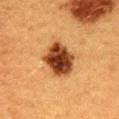The lesion was tiled from a total-body skin photograph and was not biopsied. Automated tile analysis of the lesion measured a lesion area of about 14 mm², a shape eccentricity near 0.55, and a shape-asymmetry score of about 0.15 (0 = symmetric). It also reported a border-irregularity index near 2/10 and internal color variation of about 6.5 on a 0–10 scale. The analysis additionally found a nevus-likeness score of about 100/100 and a detector confidence of about 100 out of 100 that the crop contains a lesion. Cropped from a whole-body photographic skin survey; the tile spans about 15 mm. The lesion is on the mid back. Captured under cross-polarized illumination. A female patient approximately 40 years of age. Longest diameter approximately 4.5 mm.